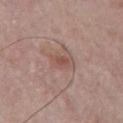Imaged during a routine full-body skin examination; the lesion was not biopsied and no histopathology is available.
Cropped from a total-body skin-imaging series; the visible field is about 15 mm.
On the chest.
The total-body-photography lesion software estimated a shape-asymmetry score of about 0.3 (0 = symmetric). And it measured an average lesion color of about L≈51 a*≈21 b*≈24 (CIELAB), a lesion–skin lightness drop of about 7, and a normalized lesion–skin contrast near 6. And it measured a color-variation rating of about 1.5/10 and radial color variation of about 0.5. It also reported an automated nevus-likeness rating near 5 out of 100.
This is a white-light tile.
The recorded lesion diameter is about 3 mm.
The patient is a male aged around 65.